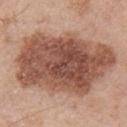Clinical impression:
Part of a total-body skin-imaging series; this lesion was reviewed on a skin check and was not flagged for biopsy.
Acquisition and patient details:
The tile uses white-light illumination. A male patient, aged around 55. Cropped from a whole-body photographic skin survey; the tile spans about 15 mm. Measured at roughly 11.5 mm in maximum diameter. The lesion is on the back.